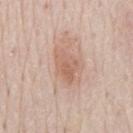A male subject, roughly 80 years of age. Measured at roughly 4 mm in maximum diameter. A region of skin cropped from a whole-body photographic capture, roughly 15 mm wide. Automated image analysis of the tile measured a lesion area of about 7.5 mm², an eccentricity of roughly 0.75, and a shape-asymmetry score of about 0.3 (0 = symmetric). The software also gave border irregularity of about 3 on a 0–10 scale, internal color variation of about 3.5 on a 0–10 scale, and a peripheral color-asymmetry measure near 1.5. The software also gave a classifier nevus-likeness of about 40/100 and a lesion-detection confidence of about 100/100. Captured under white-light illumination. From the mid back.